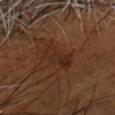Clinical impression: The lesion was photographed on a routine skin check and not biopsied; there is no pathology result. Acquisition and patient details: This image is a 15 mm lesion crop taken from a total-body photograph. Longest diameter approximately 4 mm. The patient is a male in their mid-50s. Captured under cross-polarized illumination. The lesion is on the head or neck. The total-body-photography lesion software estimated a lesion area of about 8.5 mm², an eccentricity of roughly 0.75, and a symmetry-axis asymmetry near 0.25. The analysis additionally found a nevus-likeness score of about 5/100.